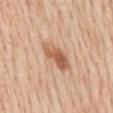Clinical impression: No biopsy was performed on this lesion — it was imaged during a full skin examination and was not determined to be concerning. Image and clinical context: This is a white-light tile. A lesion tile, about 15 mm wide, cut from a 3D total-body photograph. On the mid back. Approximately 4.5 mm at its widest. The subject is a male about 60 years old. The lesion-visualizer software estimated a footprint of about 8.5 mm², an eccentricity of roughly 0.85, and two-axis asymmetry of about 0.2. It also reported a border-irregularity rating of about 2/10 and radial color variation of about 2.5. The software also gave a nevus-likeness score of about 90/100.